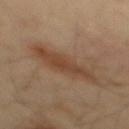{"biopsy_status": "not biopsied; imaged during a skin examination", "patient": {"sex": "male", "age_approx": 40}, "lesion_size": {"long_diameter_mm_approx": 7.0}, "site": "mid back", "automated_metrics": {"area_mm2_approx": 14.0, "eccentricity": 0.9, "shape_asymmetry": 0.25, "color_variation_0_10": 3.5, "peripheral_color_asymmetry": 1.0, "nevus_likeness_0_100": 85, "lesion_detection_confidence_0_100": 100}, "image": {"source": "total-body photography crop", "field_of_view_mm": 15}}